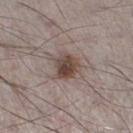Impression:
No biopsy was performed on this lesion — it was imaged during a full skin examination and was not determined to be concerning.
Image and clinical context:
A region of skin cropped from a whole-body photographic capture, roughly 15 mm wide. Measured at roughly 3.5 mm in maximum diameter. The lesion is on the left lower leg. A male subject aged 68 to 72. Imaged with white-light lighting.